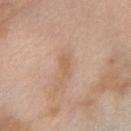Captured during whole-body skin photography for melanoma surveillance; the lesion was not biopsied.
A male subject approximately 55 years of age.
Cropped from a total-body skin-imaging series; the visible field is about 15 mm.
The lesion is on the right forearm.
The total-body-photography lesion software estimated roughly 6 lightness units darker than nearby skin and a normalized border contrast of about 5.5.
Captured under cross-polarized illumination.